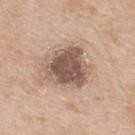Acquisition and patient details: A male subject, in their mid-60s. The tile uses white-light illumination. The total-body-photography lesion software estimated a border-irregularity index near 3/10 and radial color variation of about 1.5. A 15 mm close-up tile from a total-body photography series done for melanoma screening. On the upper back.